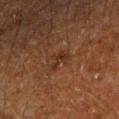biopsy status: imaged on a skin check; not biopsied | acquisition: 15 mm crop, total-body photography | lesion diameter: about 2.5 mm | location: the arm | subject: male, in their 60s | TBP lesion metrics: a footprint of about 3.5 mm² and a shape-asymmetry score of about 0.5 (0 = symmetric); a lesion color around L≈23 a*≈17 b*≈23 in CIELAB and about 5 CIELAB-L* units darker than the surrounding skin; a border-irregularity index near 5.5/10, a color-variation rating of about 1/10, and a peripheral color-asymmetry measure near 0.5; a classifier nevus-likeness of about 25/100.